Automated image analysis of the tile measured an outline eccentricity of about 0.7 (0 = round, 1 = elongated). The software also gave a mean CIELAB color near L≈38 a*≈17 b*≈18 and a lesion-to-skin contrast of about 17 (normalized; higher = more distinct). And it measured border irregularity of about 1 on a 0–10 scale, a color-variation rating of about 9.5/10, and a peripheral color-asymmetry measure near 3. The analysis additionally found a nevus-likeness score of about 0/100. A 15 mm close-up extracted from a 3D total-body photography capture. The tile uses white-light illumination. The lesion's longest dimension is about 5.5 mm. A male subject aged approximately 55 — 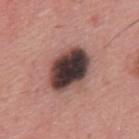Conclusion:
Histopathological examination showed a dysplastic (Clark) nevus.